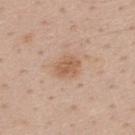<case>
<biopsy_status>not biopsied; imaged during a skin examination</biopsy_status>
<lesion_size>
  <long_diameter_mm_approx>3.0</long_diameter_mm_approx>
</lesion_size>
<automated_metrics>
  <nevus_likeness_0_100>40</nevus_likeness_0_100>
  <lesion_detection_confidence_0_100>100</lesion_detection_confidence_0_100>
</automated_metrics>
<patient>
  <sex>male</sex>
  <age_approx>40</age_approx>
</patient>
<site>upper back</site>
<image>
  <source>total-body photography crop</source>
  <field_of_view_mm>15</field_of_view_mm>
</image>
</case>